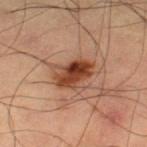  biopsy_status: not biopsied; imaged during a skin examination
  lighting: cross-polarized
  site: right thigh
  image:
    source: total-body photography crop
    field_of_view_mm: 15
  patient:
    sex: male
    age_approx: 60
  lesion_size:
    long_diameter_mm_approx: 4.5